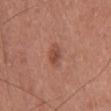Impression: Imaged during a routine full-body skin examination; the lesion was not biopsied and no histopathology is available. Background: From the chest. A 15 mm close-up extracted from a 3D total-body photography capture. An algorithmic analysis of the crop reported an area of roughly 4 mm², a shape eccentricity near 0.75, and two-axis asymmetry of about 0.25. Longest diameter approximately 3 mm. A female subject, aged 38 to 42.